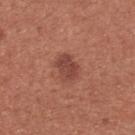follow-up = total-body-photography surveillance lesion; no biopsy | automated metrics = an area of roughly 6 mm², an eccentricity of roughly 0.65, and two-axis asymmetry of about 0.2; a border-irregularity index near 2/10 and a within-lesion color-variation index near 2/10; a lesion-detection confidence of about 100/100 | lesion diameter = ~3 mm (longest diameter) | image = ~15 mm tile from a whole-body skin photo | patient = female, aged 33–37 | body site = the chest | illumination = white-light.